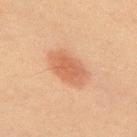Q: Was this lesion biopsied?
A: catalogued during a skin exam; not biopsied
Q: What is the anatomic site?
A: the upper back
Q: Automated lesion metrics?
A: a color-variation rating of about 2/10 and a peripheral color-asymmetry measure near 0.5; a classifier nevus-likeness of about 100/100 and lesion-presence confidence of about 100/100
Q: Illumination type?
A: cross-polarized
Q: What is the imaging modality?
A: ~15 mm crop, total-body skin-cancer survey
Q: Patient demographics?
A: male, approximately 60 years of age